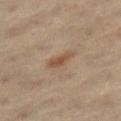{"biopsy_status": "not biopsied; imaged during a skin examination"}